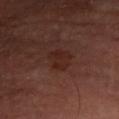Notes:
* biopsy status — no biopsy performed (imaged during a skin exam)
* lesion size — ~3 mm (longest diameter)
* TBP lesion metrics — an average lesion color of about L≈25 a*≈21 b*≈23 (CIELAB), a lesion–skin lightness drop of about 6, and a normalized border contrast of about 6.5; a border-irregularity rating of about 3/10 and internal color variation of about 2 on a 0–10 scale
* tile lighting — cross-polarized illumination
* image — total-body-photography crop, ~15 mm field of view
* location — the arm